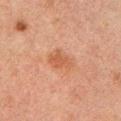biopsy status = no biopsy performed (imaged during a skin exam); site = the left upper arm; subject = male, approximately 50 years of age; imaging modality = 15 mm crop, total-body photography.